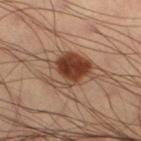The lesion was tiled from a total-body skin photograph and was not biopsied. A lesion tile, about 15 mm wide, cut from a 3D total-body photograph. Longest diameter approximately 5.5 mm. Located on the leg. Captured under cross-polarized illumination. A male subject, aged around 40.